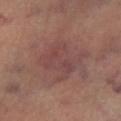{
  "biopsy_status": "not biopsied; imaged during a skin examination",
  "image": {
    "source": "total-body photography crop",
    "field_of_view_mm": 15
  },
  "lighting": "cross-polarized",
  "site": "left lower leg",
  "lesion_size": {
    "long_diameter_mm_approx": 5.0
  },
  "patient": {
    "age_approx": 65
  }
}